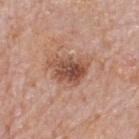Captured during whole-body skin photography for melanoma surveillance; the lesion was not biopsied. A close-up tile cropped from a whole-body skin photograph, about 15 mm across. On the upper back. A male subject in their mid- to late 70s.